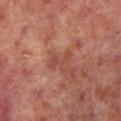Part of a total-body skin-imaging series; this lesion was reviewed on a skin check and was not flagged for biopsy. A 15 mm crop from a total-body photograph taken for skin-cancer surveillance. Measured at roughly 3 mm in maximum diameter. The patient is a male roughly 65 years of age. The lesion is on the left lower leg. Imaged with cross-polarized lighting.